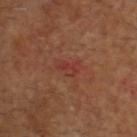Q: Was a biopsy performed?
A: total-body-photography surveillance lesion; no biopsy
Q: Lesion location?
A: the head or neck
Q: How was the tile lit?
A: cross-polarized illumination
Q: What are the patient's age and sex?
A: male, approximately 60 years of age
Q: How was this image acquired?
A: ~15 mm crop, total-body skin-cancer survey